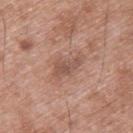Imaged during a routine full-body skin examination; the lesion was not biopsied and no histopathology is available.
Approximately 4 mm at its widest.
The tile uses white-light illumination.
A male subject, aged approximately 50.
Automated tile analysis of the lesion measured a lesion–skin lightness drop of about 9 and a lesion-to-skin contrast of about 6 (normalized; higher = more distinct). It also reported a border-irregularity rating of about 4.5/10 and peripheral color asymmetry of about 1. The analysis additionally found a classifier nevus-likeness of about 0/100 and a detector confidence of about 100 out of 100 that the crop contains a lesion.
Cropped from a whole-body photographic skin survey; the tile spans about 15 mm.
Located on the upper back.